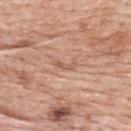A female subject, aged around 60. Longest diameter approximately 2.5 mm. Imaged with white-light lighting. The lesion is on the upper back. A region of skin cropped from a whole-body photographic capture, roughly 15 mm wide.